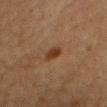The lesion was photographed on a routine skin check and not biopsied; there is no pathology result.
Automated image analysis of the tile measured an area of roughly 3.5 mm², an outline eccentricity of about 0.75 (0 = round, 1 = elongated), and two-axis asymmetry of about 0.25. And it measured an average lesion color of about L≈31 a*≈18 b*≈29 (CIELAB) and a normalized lesion–skin contrast near 8.5. The software also gave a border-irregularity index near 2/10, a within-lesion color-variation index near 3/10, and a peripheral color-asymmetry measure near 1. The software also gave lesion-presence confidence of about 100/100.
The subject is a male aged around 60.
Longest diameter approximately 2.5 mm.
The lesion is located on the chest.
A 15 mm crop from a total-body photograph taken for skin-cancer surveillance.
Imaged with cross-polarized lighting.